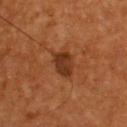{
  "biopsy_status": "not biopsied; imaged during a skin examination",
  "site": "upper back",
  "image": {
    "source": "total-body photography crop",
    "field_of_view_mm": 15
  },
  "patient": {
    "sex": "male",
    "age_approx": 55
  },
  "lesion_size": {
    "long_diameter_mm_approx": 3.5
  }
}